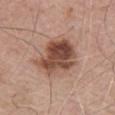| feature | finding |
|---|---|
| biopsy status | total-body-photography surveillance lesion; no biopsy |
| location | the chest |
| lighting | white-light illumination |
| image | ~15 mm tile from a whole-body skin photo |
| automated metrics | an average lesion color of about L≈48 a*≈21 b*≈27 (CIELAB) and a normalized border contrast of about 11; a border-irregularity rating of about 3/10, a within-lesion color-variation index near 7/10, and a peripheral color-asymmetry measure near 2.5; a classifier nevus-likeness of about 65/100 and a detector confidence of about 100 out of 100 that the crop contains a lesion |
| subject | male, aged around 65 |